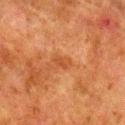Captured under cross-polarized illumination.
A 15 mm crop from a total-body photograph taken for skin-cancer surveillance.
The lesion is located on the right lower leg.
The lesion's longest dimension is about 2.5 mm.
The lesion-visualizer software estimated a mean CIELAB color near L≈40 a*≈25 b*≈35 and about 6 CIELAB-L* units darker than the surrounding skin. The analysis additionally found border irregularity of about 3.5 on a 0–10 scale and a within-lesion color-variation index near 0/10.
A male patient aged 78 to 82.